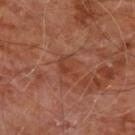Captured during whole-body skin photography for melanoma surveillance; the lesion was not biopsied.
The lesion's longest dimension is about 3 mm.
Automated tile analysis of the lesion measured a mean CIELAB color near L≈39 a*≈25 b*≈30, a lesion–skin lightness drop of about 6, and a normalized lesion–skin contrast near 5.5. The analysis additionally found border irregularity of about 3 on a 0–10 scale, internal color variation of about 3 on a 0–10 scale, and radial color variation of about 1.
Captured under cross-polarized illumination.
The lesion is on the chest.
A 15 mm close-up tile from a total-body photography series done for melanoma screening.
A male subject, aged approximately 60.